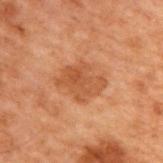{
  "biopsy_status": "not biopsied; imaged during a skin examination",
  "lighting": "cross-polarized",
  "patient": {
    "sex": "male",
    "age_approx": 70
  },
  "automated_metrics": {
    "area_mm2_approx": 11.0,
    "eccentricity": 0.65,
    "cielab_L": 40,
    "cielab_a": 21,
    "cielab_b": 31,
    "vs_skin_darker_L": 7.0,
    "vs_skin_contrast_norm": 6.5,
    "border_irregularity_0_10": 4.0,
    "color_variation_0_10": 3.0
  },
  "site": "back",
  "image": {
    "source": "total-body photography crop",
    "field_of_view_mm": 15
  }
}